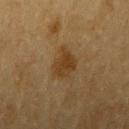<tbp_lesion>
<biopsy_status>not biopsied; imaged during a skin examination</biopsy_status>
<patient>
  <sex>male</sex>
  <age_approx>85+</age_approx>
</patient>
<lighting>cross-polarized</lighting>
<lesion_size>
  <long_diameter_mm_approx>3.5</long_diameter_mm_approx>
</lesion_size>
<image>
  <source>total-body photography crop</source>
  <field_of_view_mm>15</field_of_view_mm>
</image>
<site>right upper arm</site>
</tbp_lesion>